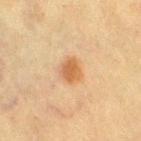Assessment:
The lesion was tiled from a total-body skin photograph and was not biopsied.